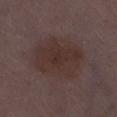Q: Was this lesion biopsied?
A: catalogued during a skin exam; not biopsied
Q: What kind of image is this?
A: ~15 mm tile from a whole-body skin photo
Q: Lesion location?
A: the right thigh
Q: Illumination type?
A: white-light
Q: Lesion size?
A: ≈6 mm
Q: What did automated image analysis measure?
A: an average lesion color of about L≈31 a*≈15 b*≈18 (CIELAB), about 6 CIELAB-L* units darker than the surrounding skin, and a normalized border contrast of about 7; border irregularity of about 2.5 on a 0–10 scale and peripheral color asymmetry of about 1; a nevus-likeness score of about 10/100
Q: Patient demographics?
A: female, aged around 30